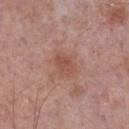Q: Was this lesion biopsied?
A: no biopsy performed (imaged during a skin exam)
Q: What is the imaging modality?
A: ~15 mm crop, total-body skin-cancer survey
Q: Where on the body is the lesion?
A: the chest
Q: What are the patient's age and sex?
A: male, about 75 years old